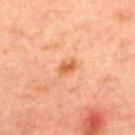This lesion was catalogued during total-body skin photography and was not selected for biopsy. From the upper back. A 15 mm crop from a total-body photograph taken for skin-cancer surveillance. Automated image analysis of the tile measured a lesion color around L≈61 a*≈28 b*≈41 in CIELAB, roughly 11 lightness units darker than nearby skin, and a lesion-to-skin contrast of about 7.5 (normalized; higher = more distinct). The software also gave border irregularity of about 2.5 on a 0–10 scale and peripheral color asymmetry of about 1. A female patient, aged approximately 45. This is a cross-polarized tile.